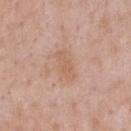follow-up = total-body-photography surveillance lesion; no biopsy | imaging modality = ~15 mm crop, total-body skin-cancer survey | patient = male, aged around 55 | image-analysis metrics = border irregularity of about 3.5 on a 0–10 scale, a within-lesion color-variation index near 1/10, and peripheral color asymmetry of about 0.5; a detector confidence of about 100 out of 100 that the crop contains a lesion | body site = the chest | tile lighting = white-light illumination.